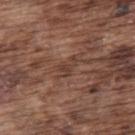<record>
  <biopsy_status>not biopsied; imaged during a skin examination</biopsy_status>
  <lighting>white-light</lighting>
  <patient>
    <sex>male</sex>
    <age_approx>75</age_approx>
  </patient>
  <site>back</site>
  <image>
    <source>total-body photography crop</source>
    <field_of_view_mm>15</field_of_view_mm>
  </image>
  <lesion_size>
    <long_diameter_mm_approx>2.5</long_diameter_mm_approx>
  </lesion_size>
  <automated_metrics>
    <border_irregularity_0_10>7.0</border_irregularity_0_10>
    <color_variation_0_10>0.0</color_variation_0_10>
    <peripheral_color_asymmetry>0.0</peripheral_color_asymmetry>
    <nevus_likeness_0_100>0</nevus_likeness_0_100>
    <lesion_detection_confidence_0_100>75</lesion_detection_confidence_0_100>
  </automated_metrics>
</record>